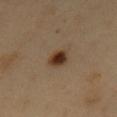Recorded during total-body skin imaging; not selected for excision or biopsy.
Cropped from a total-body skin-imaging series; the visible field is about 15 mm.
The lesion-visualizer software estimated a mean CIELAB color near L≈35 a*≈17 b*≈28, about 13 CIELAB-L* units darker than the surrounding skin, and a lesion-to-skin contrast of about 12 (normalized; higher = more distinct). The software also gave a border-irregularity rating of about 2/10, internal color variation of about 5 on a 0–10 scale, and peripheral color asymmetry of about 1.5.
On the chest.
Longest diameter approximately 3 mm.
A male subject, aged approximately 50.
Captured under cross-polarized illumination.